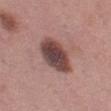  site: left thigh
  patient:
    sex: female
    age_approx: 50
  image:
    source: total-body photography crop
    field_of_view_mm: 15